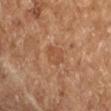Notes:
- notes: total-body-photography surveillance lesion; no biopsy
- automated metrics: an area of roughly 4.5 mm² and a shape-asymmetry score of about 0.25 (0 = symmetric); a mean CIELAB color near L≈48 a*≈22 b*≈33, roughly 6 lightness units darker than nearby skin, and a lesion-to-skin contrast of about 5 (normalized; higher = more distinct); a border-irregularity rating of about 2.5/10, internal color variation of about 2.5 on a 0–10 scale, and radial color variation of about 1
- subject: female, aged 68 to 72
- illumination: cross-polarized
- size: ≈2.5 mm
- acquisition: 15 mm crop, total-body photography
- site: the right forearm